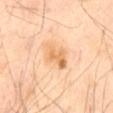Clinical impression:
Part of a total-body skin-imaging series; this lesion was reviewed on a skin check and was not flagged for biopsy.
Clinical summary:
Cropped from a whole-body photographic skin survey; the tile spans about 15 mm. This is a cross-polarized tile. About 4 mm across. The lesion is on the front of the torso. Automated image analysis of the tile measured an area of roughly 7.5 mm², an outline eccentricity of about 0.8 (0 = round, 1 = elongated), and a shape-asymmetry score of about 0.35 (0 = symmetric). The analysis additionally found a mean CIELAB color near L≈70 a*≈21 b*≈41, roughly 10 lightness units darker than nearby skin, and a normalized border contrast of about 7. The analysis additionally found border irregularity of about 3.5 on a 0–10 scale, a within-lesion color-variation index near 5.5/10, and a peripheral color-asymmetry measure near 2. It also reported a nevus-likeness score of about 5/100 and lesion-presence confidence of about 100/100. A male patient, in their 70s.